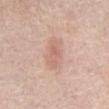{"biopsy_status": "not biopsied; imaged during a skin examination", "patient": {"sex": "male", "age_approx": 60}, "image": {"source": "total-body photography crop", "field_of_view_mm": 15}, "lesion_size": {"long_diameter_mm_approx": 3.5}, "site": "abdomen", "automated_metrics": {"area_mm2_approx": 5.0, "eccentricity": 0.85, "shape_asymmetry": 0.2, "border_irregularity_0_10": 2.5, "color_variation_0_10": 3.0, "peripheral_color_asymmetry": 1.0}}